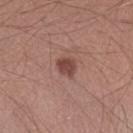Assessment:
Part of a total-body skin-imaging series; this lesion was reviewed on a skin check and was not flagged for biopsy.
Clinical summary:
Imaged with white-light lighting. A region of skin cropped from a whole-body photographic capture, roughly 15 mm wide. The recorded lesion diameter is about 2.5 mm. The patient is a male about 25 years old. From the left forearm.